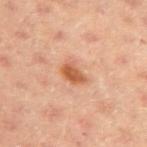biopsy status: imaged on a skin check; not biopsied | automated metrics: a lesion area of about 5 mm² and two-axis asymmetry of about 0.25; a nevus-likeness score of about 95/100 and a detector confidence of about 100 out of 100 that the crop contains a lesion | subject: female, aged approximately 40 | location: the right upper arm | lesion diameter: about 3 mm | acquisition: 15 mm crop, total-body photography.